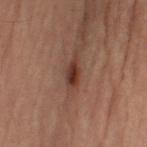No biopsy was performed on this lesion — it was imaged during a full skin examination and was not determined to be concerning.
A 15 mm close-up tile from a total-body photography series done for melanoma screening.
The lesion is located on the left upper arm.
The subject is a male aged approximately 60.
The lesion's longest dimension is about 3 mm.
An algorithmic analysis of the crop reported an outline eccentricity of about 0.85 (0 = round, 1 = elongated). It also reported border irregularity of about 2.5 on a 0–10 scale, a color-variation rating of about 4/10, and radial color variation of about 1.5. The analysis additionally found an automated nevus-likeness rating near 90 out of 100 and lesion-presence confidence of about 100/100.